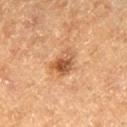follow-up: imaged on a skin check; not biopsied
location: the left thigh
lighting: cross-polarized illumination
diameter: ≈3.5 mm
subject: male, approximately 75 years of age
acquisition: 15 mm crop, total-body photography